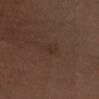Q: Was this lesion biopsied?
A: no biopsy performed (imaged during a skin exam)
Q: Patient demographics?
A: female, about 50 years old
Q: How was the tile lit?
A: white-light
Q: What is the anatomic site?
A: the head or neck
Q: How was this image acquired?
A: ~15 mm crop, total-body skin-cancer survey
Q: Automated lesion metrics?
A: an outline eccentricity of about 0.65 (0 = round, 1 = elongated) and a shape-asymmetry score of about 0.3 (0 = symmetric); a lesion color around L≈29 a*≈17 b*≈24 in CIELAB, roughly 4 lightness units darker than nearby skin, and a normalized lesion–skin contrast near 5; a within-lesion color-variation index near 0/10 and a peripheral color-asymmetry measure near 0